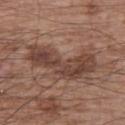| field | value |
|---|---|
| biopsy status | total-body-photography surveillance lesion; no biopsy |
| lighting | white-light |
| lesion diameter | about 8.5 mm |
| image source | total-body-photography crop, ~15 mm field of view |
| patient | male, aged approximately 65 |
| location | the left upper arm |
| image-analysis metrics | border irregularity of about 6.5 on a 0–10 scale and radial color variation of about 1.5 |